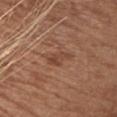The lesion was tiled from a total-body skin photograph and was not biopsied. The tile uses white-light illumination. Cropped from a whole-body photographic skin survey; the tile spans about 15 mm. The lesion is located on the chest. Approximately 3.5 mm at its widest. A female subject aged 38 to 42.